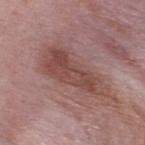| field | value |
|---|---|
| workup | imaged on a skin check; not biopsied |
| size | ≈8 mm |
| anatomic site | the upper back |
| patient | male, approximately 50 years of age |
| image source | ~15 mm crop, total-body skin-cancer survey |
| automated metrics | a lesion–skin lightness drop of about 9 and a lesion-to-skin contrast of about 7.5 (normalized; higher = more distinct); border irregularity of about 4 on a 0–10 scale and a color-variation rating of about 4/10; a lesion-detection confidence of about 100/100 |
| illumination | white-light illumination |